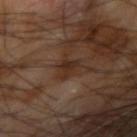follow-up: total-body-photography surveillance lesion; no biopsy | imaging modality: 15 mm crop, total-body photography | image-analysis metrics: a footprint of about 4.5 mm² and an eccentricity of roughly 0.5; border irregularity of about 3 on a 0–10 scale; a detector confidence of about 100 out of 100 that the crop contains a lesion | patient: male, aged 63–67 | location: the right upper arm.